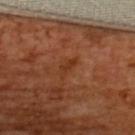This lesion was catalogued during total-body skin photography and was not selected for biopsy. A 15 mm crop from a total-body photograph taken for skin-cancer surveillance. From the upper back. A female subject, in their mid-50s.